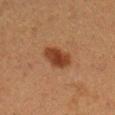follow-up: no biopsy performed (imaged during a skin exam); image source: 15 mm crop, total-body photography; anatomic site: the right lower leg; patient: female, roughly 40 years of age.